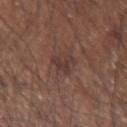| key | value |
|---|---|
| follow-up | no biopsy performed (imaged during a skin exam) |
| lighting | white-light |
| subject | male, in their mid- to late 60s |
| acquisition | ~15 mm crop, total-body skin-cancer survey |
| lesion size | ~3.5 mm (longest diameter) |
| location | the right upper arm |